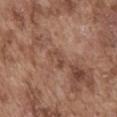notes = catalogued during a skin exam; not biopsied.